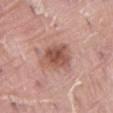biopsy status = catalogued during a skin exam; not biopsied
image source = total-body-photography crop, ~15 mm field of view
anatomic site = the abdomen
subject = male, in their 40s
size = about 4 mm